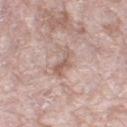<lesion>
<lesion_size>
  <long_diameter_mm_approx>3.5</long_diameter_mm_approx>
</lesion_size>
<site>right thigh</site>
<lighting>white-light</lighting>
<image>
  <source>total-body photography crop</source>
  <field_of_view_mm>15</field_of_view_mm>
</image>
<patient>
  <sex>female</sex>
  <age_approx>70</age_approx>
</patient>
<automated_metrics>
  <area_mm2_approx>4.5</area_mm2_approx>
  <eccentricity>0.9</eccentricity>
  <nevus_likeness_0_100>0</nevus_likeness_0_100>
  <lesion_detection_confidence_0_100>95</lesion_detection_confidence_0_100>
</automated_metrics>
</lesion>